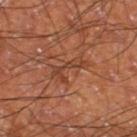<lesion>
  <biopsy_status>not biopsied; imaged during a skin examination</biopsy_status>
  <image>
    <source>total-body photography crop</source>
    <field_of_view_mm>15</field_of_view_mm>
  </image>
  <automated_metrics>
    <cielab_L>38</cielab_L>
    <cielab_a>22</cielab_a>
    <cielab_b>29</cielab_b>
    <vs_skin_darker_L>7.0</vs_skin_darker_L>
    <vs_skin_contrast_norm>6.0</vs_skin_contrast_norm>
    <color_variation_0_10>3.5</color_variation_0_10>
    <peripheral_color_asymmetry>1.0</peripheral_color_asymmetry>
  </automated_metrics>
  <lesion_size>
    <long_diameter_mm_approx>4.5</long_diameter_mm_approx>
  </lesion_size>
  <site>left thigh</site>
  <patient>
    <sex>male</sex>
    <age_approx>60</age_approx>
  </patient>
</lesion>